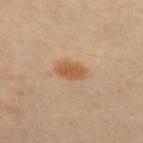biopsy status = no biopsy performed (imaged during a skin exam) | TBP lesion metrics = border irregularity of about 1.5 on a 0–10 scale, a color-variation rating of about 1.5/10, and a peripheral color-asymmetry measure near 0.5; a nevus-likeness score of about 95/100 and lesion-presence confidence of about 100/100 | image = ~15 mm crop, total-body skin-cancer survey | body site = the mid back | subject = female, in their 30s.